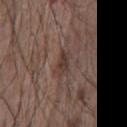Impression:
This lesion was catalogued during total-body skin photography and was not selected for biopsy.
Acquisition and patient details:
Automated tile analysis of the lesion measured a lesion area of about 4.5 mm² and an eccentricity of roughly 0.85. It also reported a mean CIELAB color near L≈37 a*≈15 b*≈21. The software also gave a within-lesion color-variation index near 3/10 and peripheral color asymmetry of about 1. And it measured an automated nevus-likeness rating near 0 out of 100. A male patient, aged approximately 55. Cropped from a whole-body photographic skin survey; the tile spans about 15 mm. Imaged with white-light lighting. Located on the chest. Approximately 3 mm at its widest.